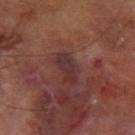Captured during whole-body skin photography for melanoma surveillance; the lesion was not biopsied.
Measured at roughly 4 mm in maximum diameter.
Automated image analysis of the tile measured border irregularity of about 3 on a 0–10 scale, internal color variation of about 3.5 on a 0–10 scale, and radial color variation of about 1. The software also gave an automated nevus-likeness rating near 0 out of 100 and lesion-presence confidence of about 95/100.
A male patient, in their mid-60s.
A close-up tile cropped from a whole-body skin photograph, about 15 mm across.
On the arm.